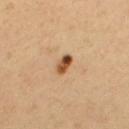notes=catalogued during a skin exam; not biopsied
body site=the upper back
image=~15 mm tile from a whole-body skin photo
subject=male, aged approximately 40